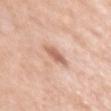Q: Was this lesion biopsied?
A: imaged on a skin check; not biopsied
Q: Lesion location?
A: the right upper arm
Q: What kind of image is this?
A: total-body-photography crop, ~15 mm field of view
Q: Who is the patient?
A: female, aged 53–57